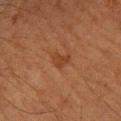| field | value |
|---|---|
| TBP lesion metrics | a border-irregularity index near 4/10 and internal color variation of about 1 on a 0–10 scale |
| patient | male, roughly 50 years of age |
| image source | ~15 mm crop, total-body skin-cancer survey |
| body site | the right forearm |